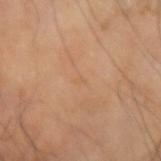follow-up = total-body-photography surveillance lesion; no biopsy
image source = ~15 mm tile from a whole-body skin photo
subject = male, aged around 65
lighting = cross-polarized
automated lesion analysis = a lesion area of about 0.5 mm²; lesion-presence confidence of about 100/100
lesion diameter = ≈1 mm
body site = the left forearm